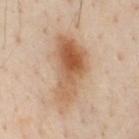Case summary:
• follow-up · total-body-photography surveillance lesion; no biopsy
• body site · the chest
• subject · male, in their mid- to late 50s
• imaging modality · total-body-photography crop, ~15 mm field of view